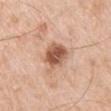– follow-up · imaged on a skin check; not biopsied